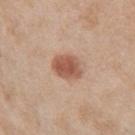biopsy status: total-body-photography surveillance lesion; no biopsy | automated lesion analysis: an area of roughly 8 mm², an outline eccentricity of about 0.55 (0 = round, 1 = elongated), and a symmetry-axis asymmetry near 0.2; a border-irregularity index near 1.5/10, a within-lesion color-variation index near 3/10, and peripheral color asymmetry of about 1; an automated nevus-likeness rating near 100 out of 100 and a lesion-detection confidence of about 100/100 | patient: male, approximately 65 years of age | location: the right upper arm | tile lighting: white-light illumination | image source: ~15 mm tile from a whole-body skin photo.